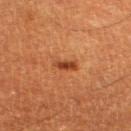Case summary:
- patient — male, aged around 60
- site — the left thigh
- lighting — cross-polarized
- image — 15 mm crop, total-body photography